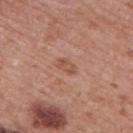Notes:
- notes · no biopsy performed (imaged during a skin exam)
- patient · male, about 65 years old
- image-analysis metrics · a lesion area of about 3 mm², an outline eccentricity of about 0.85 (0 = round, 1 = elongated), and a shape-asymmetry score of about 0.3 (0 = symmetric); a mean CIELAB color near L≈52 a*≈22 b*≈30, about 8 CIELAB-L* units darker than the surrounding skin, and a normalized lesion–skin contrast near 6; a border-irregularity index near 3/10 and a color-variation rating of about 2.5/10
- image · ~15 mm crop, total-body skin-cancer survey
- body site · the upper back
- lesion size · ~2.5 mm (longest diameter)